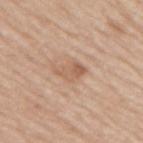Q: Was this lesion biopsied?
A: catalogued during a skin exam; not biopsied
Q: Lesion location?
A: the upper back
Q: How large is the lesion?
A: ~3.5 mm (longest diameter)
Q: What is the imaging modality?
A: ~15 mm crop, total-body skin-cancer survey
Q: Illumination type?
A: white-light
Q: What are the patient's age and sex?
A: male, aged approximately 65
Q: Automated lesion metrics?
A: an automated nevus-likeness rating near 10 out of 100